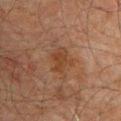Q: Was this lesion biopsied?
A: catalogued during a skin exam; not biopsied
Q: Patient demographics?
A: male, aged 58 to 62
Q: What lighting was used for the tile?
A: cross-polarized illumination
Q: What did automated image analysis measure?
A: a footprint of about 6 mm², an eccentricity of roughly 0.65, and two-axis asymmetry of about 0.3; about 6 CIELAB-L* units darker than the surrounding skin and a normalized border contrast of about 6.5; a border-irregularity rating of about 4/10 and a within-lesion color-variation index near 2/10; an automated nevus-likeness rating near 0 out of 100
Q: How was this image acquired?
A: ~15 mm tile from a whole-body skin photo
Q: What is the anatomic site?
A: the front of the torso